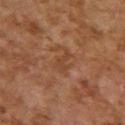| key | value |
|---|---|
| anatomic site | the upper back |
| imaging modality | ~15 mm crop, total-body skin-cancer survey |
| size | about 3 mm |
| illumination | cross-polarized |
| TBP lesion metrics | an area of roughly 4 mm², an eccentricity of roughly 0.8, and two-axis asymmetry of about 0.45; a nevus-likeness score of about 0/100 and lesion-presence confidence of about 100/100 |
| patient | female, about 60 years old |